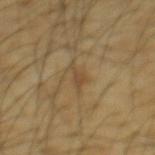workup = catalogued during a skin exam; not biopsied | location = the mid back | size = ~3 mm (longest diameter) | image = 15 mm crop, total-body photography | image-analysis metrics = an eccentricity of roughly 0.9 and two-axis asymmetry of about 0.5 | lighting = cross-polarized illumination | patient = male, aged 63 to 67.